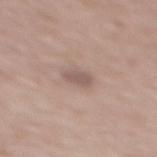Part of a total-body skin-imaging series; this lesion was reviewed on a skin check and was not flagged for biopsy.
The total-body-photography lesion software estimated an average lesion color of about L≈55 a*≈16 b*≈22 (CIELAB), a lesion–skin lightness drop of about 9, and a lesion-to-skin contrast of about 6.5 (normalized; higher = more distinct).
A female patient, in their mid- to late 60s.
A close-up tile cropped from a whole-body skin photograph, about 15 mm across.
The recorded lesion diameter is about 2.5 mm.
From the mid back.